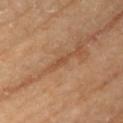image: 15 mm crop, total-body photography
subject: female, in their 70s
site: the upper back